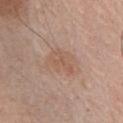Notes:
- follow-up — catalogued during a skin exam; not biopsied
- size — ≈4 mm
- image source — ~15 mm crop, total-body skin-cancer survey
- tile lighting — white-light
- patient — male, aged approximately 55
- body site — the chest
- image-analysis metrics — about 6 CIELAB-L* units darker than the surrounding skin and a lesion-to-skin contrast of about 5 (normalized; higher = more distinct)